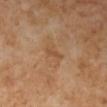| feature | finding |
|---|---|
| tile lighting | cross-polarized |
| location | the right lower leg |
| acquisition | ~15 mm tile from a whole-body skin photo |
| subject | female, aged 48–52 |
| image-analysis metrics | a mean CIELAB color near L≈51 a*≈21 b*≈35, roughly 6 lightness units darker than nearby skin, and a lesion-to-skin contrast of about 5 (normalized; higher = more distinct); a border-irregularity index near 3/10, a color-variation rating of about 0/10, and peripheral color asymmetry of about 0 |
| lesion size | ~2.5 mm (longest diameter) |